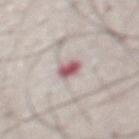| feature | finding |
|---|---|
| tile lighting | white-light illumination |
| automated lesion analysis | a footprint of about 4 mm², a shape eccentricity near 0.65, and a shape-asymmetry score of about 0.2 (0 = symmetric); an average lesion color of about L≈58 a*≈22 b*≈17 (CIELAB), roughly 16 lightness units darker than nearby skin, and a normalized lesion–skin contrast near 10; a classifier nevus-likeness of about 5/100 and a lesion-detection confidence of about 80/100 |
| site | the abdomen |
| imaging modality | total-body-photography crop, ~15 mm field of view |
| subject | male, in their 50s |